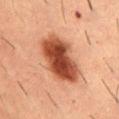Findings:
- notes · no biopsy performed (imaged during a skin exam)
- anatomic site · the lower back
- imaging modality · total-body-photography crop, ~15 mm field of view
- illumination · cross-polarized illumination
- automated lesion analysis · a footprint of about 21 mm², an outline eccentricity of about 0.85 (0 = round, 1 = elongated), and a symmetry-axis asymmetry near 0.2; a classifier nevus-likeness of about 100/100 and lesion-presence confidence of about 100/100
- subject · male, aged 53–57